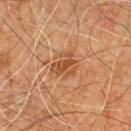Q: Was a biopsy performed?
A: no biopsy performed (imaged during a skin exam)
Q: What are the patient's age and sex?
A: male, aged approximately 60
Q: Lesion location?
A: the front of the torso
Q: What is the imaging modality?
A: 15 mm crop, total-body photography